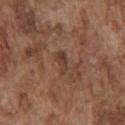biopsy status: catalogued during a skin exam; not biopsied | location: the chest | illumination: white-light illumination | subject: male, in their mid-70s | image source: ~15 mm crop, total-body skin-cancer survey.